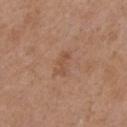Recorded during total-body skin imaging; not selected for excision or biopsy.
Located on the chest.
A female subject, about 75 years old.
This is a white-light tile.
The recorded lesion diameter is about 2.5 mm.
A lesion tile, about 15 mm wide, cut from a 3D total-body photograph.
An algorithmic analysis of the crop reported an average lesion color of about L≈50 a*≈21 b*≈31 (CIELAB), about 7 CIELAB-L* units darker than the surrounding skin, and a lesion-to-skin contrast of about 5.5 (normalized; higher = more distinct). And it measured a within-lesion color-variation index near 0/10 and peripheral color asymmetry of about 0.